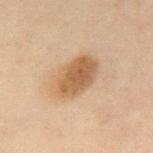Part of a total-body skin-imaging series; this lesion was reviewed on a skin check and was not flagged for biopsy. The subject is a female aged 28–32. The lesion is located on the back. Imaged with cross-polarized lighting. Automated tile analysis of the lesion measured an average lesion color of about L≈47 a*≈15 b*≈30 (CIELAB) and a normalized lesion–skin contrast near 8. A 15 mm crop from a total-body photograph taken for skin-cancer surveillance. Approximately 5.5 mm at its widest.